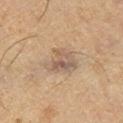Impression:
Captured during whole-body skin photography for melanoma surveillance; the lesion was not biopsied.
Clinical summary:
Imaged with cross-polarized lighting. The recorded lesion diameter is about 4 mm. The lesion is on the left lower leg. A male patient in their mid- to late 60s. A close-up tile cropped from a whole-body skin photograph, about 15 mm across.